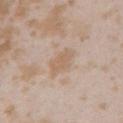  biopsy_status: not biopsied; imaged during a skin examination
  site: left upper arm
  patient:
    sex: female
    age_approx: 25
  image:
    source: total-body photography crop
    field_of_view_mm: 15